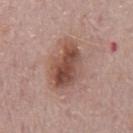<tbp_lesion>
<biopsy_status>not biopsied; imaged during a skin examination</biopsy_status>
<site>mid back</site>
<lighting>white-light</lighting>
<image>
  <source>total-body photography crop</source>
  <field_of_view_mm>15</field_of_view_mm>
</image>
<patient>
  <sex>male</sex>
  <age_approx>75</age_approx>
</patient>
<lesion_size>
  <long_diameter_mm_approx>6.0</long_diameter_mm_approx>
</lesion_size>
</tbp_lesion>